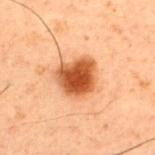<record>
<biopsy_status>not biopsied; imaged during a skin examination</biopsy_status>
<automated_metrics>
  <area_mm2_approx>14.0</area_mm2_approx>
  <shape_asymmetry>0.25</shape_asymmetry>
</automated_metrics>
<lighting>cross-polarized</lighting>
<lesion_size>
  <long_diameter_mm_approx>4.5</long_diameter_mm_approx>
</lesion_size>
<site>upper back</site>
<image>
  <source>total-body photography crop</source>
  <field_of_view_mm>15</field_of_view_mm>
</image>
<patient>
  <sex>male</sex>
  <age_approx>60</age_approx>
</patient>
</record>